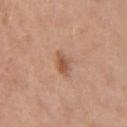The lesion is on the arm. A female subject aged around 40. A 15 mm close-up extracted from a 3D total-body photography capture.